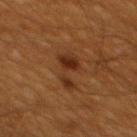Case summary:
- notes — catalogued during a skin exam; not biopsied
- patient — male, aged approximately 60
- body site — the mid back
- image source — 15 mm crop, total-body photography